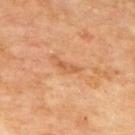Impression:
Captured during whole-body skin photography for melanoma surveillance; the lesion was not biopsied.
Image and clinical context:
A region of skin cropped from a whole-body photographic capture, roughly 15 mm wide. The lesion's longest dimension is about 3 mm. The lesion-visualizer software estimated an outline eccentricity of about 0.95 (0 = round, 1 = elongated) and a symmetry-axis asymmetry near 0.3. And it measured an average lesion color of about L≈58 a*≈25 b*≈40 (CIELAB) and a normalized lesion–skin contrast near 6. The analysis additionally found border irregularity of about 3.5 on a 0–10 scale, a within-lesion color-variation index near 0/10, and peripheral color asymmetry of about 0. Imaged with cross-polarized lighting. The patient is a male roughly 70 years of age. The lesion is located on the back.